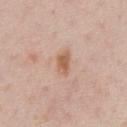No biopsy was performed on this lesion — it was imaged during a full skin examination and was not determined to be concerning. This image is a 15 mm lesion crop taken from a total-body photograph. A male subject, about 60 years old. The tile uses white-light illumination. About 3.5 mm across. The lesion is located on the chest.